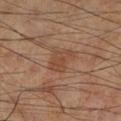Recorded during total-body skin imaging; not selected for excision or biopsy. A region of skin cropped from a whole-body photographic capture, roughly 15 mm wide. Captured under cross-polarized illumination. The subject is a male approximately 70 years of age. Measured at roughly 3.5 mm in maximum diameter. From the leg.